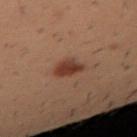Recorded during total-body skin imaging; not selected for excision or biopsy.
A region of skin cropped from a whole-body photographic capture, roughly 15 mm wide.
The patient is a male aged around 30.
From the arm.
Automated image analysis of the tile measured a symmetry-axis asymmetry near 0.25. And it measured a mean CIELAB color near L≈30 a*≈18 b*≈23, a lesion–skin lightness drop of about 9, and a normalized lesion–skin contrast near 9. The software also gave a border-irregularity rating of about 2/10 and peripheral color asymmetry of about 1. The analysis additionally found lesion-presence confidence of about 100/100.